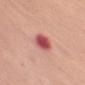Impression:
This lesion was catalogued during total-body skin photography and was not selected for biopsy.
Image and clinical context:
The tile uses white-light illumination. The lesion is on the right upper arm. A roughly 15 mm field-of-view crop from a total-body skin photograph. The patient is a female approximately 65 years of age. An algorithmic analysis of the crop reported a mean CIELAB color near L≈54 a*≈35 b*≈25, a lesion–skin lightness drop of about 16, and a normalized border contrast of about 10.5. And it measured lesion-presence confidence of about 100/100.